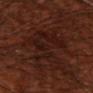follow-up = imaged on a skin check; not biopsied
image source = ~15 mm crop, total-body skin-cancer survey
subject = male, approximately 70 years of age
lighting = cross-polarized illumination
diameter = ≈8 mm
site = the right upper arm